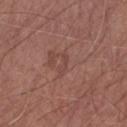Part of a total-body skin-imaging series; this lesion was reviewed on a skin check and was not flagged for biopsy. Longest diameter approximately 3 mm. A male subject, aged 63–67. Cropped from a total-body skin-imaging series; the visible field is about 15 mm. From the arm.